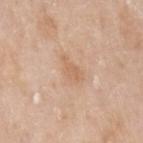This lesion was catalogued during total-body skin photography and was not selected for biopsy.
On the left upper arm.
Cropped from a whole-body photographic skin survey; the tile spans about 15 mm.
The subject is a female in their mid-50s.